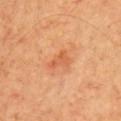Recorded during total-body skin imaging; not selected for excision or biopsy. The lesion-visualizer software estimated an average lesion color of about L≈57 a*≈28 b*≈40 (CIELAB). The software also gave a border-irregularity index near 4.5/10 and a color-variation rating of about 1/10. A male patient, roughly 70 years of age. From the chest. About 3 mm across. A region of skin cropped from a whole-body photographic capture, roughly 15 mm wide. Captured under cross-polarized illumination.